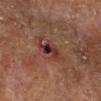The lesion was photographed on a routine skin check and not biopsied; there is no pathology result. A 15 mm close-up tile from a total-body photography series done for melanoma screening. Imaged with cross-polarized lighting. The patient is in their mid-60s. From the right lower leg. Approximately 4 mm at its widest.